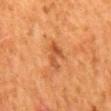Clinical impression:
The lesion was photographed on a routine skin check and not biopsied; there is no pathology result.